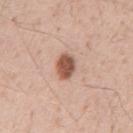Cropped from a whole-body photographic skin survey; the tile spans about 15 mm. The total-body-photography lesion software estimated a lesion area of about 6 mm² and a shape eccentricity near 0.7. The analysis additionally found a mean CIELAB color near L≈54 a*≈22 b*≈29 and about 16 CIELAB-L* units darker than the surrounding skin. The software also gave a border-irregularity rating of about 1.5/10. The patient is a male aged around 55. Captured under white-light illumination. The recorded lesion diameter is about 3.5 mm. The lesion is located on the abdomen.